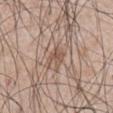Q: Was this lesion biopsied?
A: total-body-photography surveillance lesion; no biopsy
Q: Where on the body is the lesion?
A: the chest
Q: What kind of image is this?
A: total-body-photography crop, ~15 mm field of view
Q: How was the tile lit?
A: white-light
Q: Lesion size?
A: about 2.5 mm
Q: Patient demographics?
A: male, in their mid- to late 40s